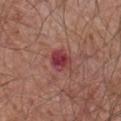Impression:
No biopsy was performed on this lesion — it was imaged during a full skin examination and was not determined to be concerning.
Image and clinical context:
The lesion is on the chest. The total-body-photography lesion software estimated an average lesion color of about L≈40 a*≈32 b*≈21 (CIELAB) and a normalized border contrast of about 9.5. And it measured a nevus-likeness score of about 0/100 and a lesion-detection confidence of about 100/100. A male patient, aged 63 to 67. The tile uses white-light illumination. A roughly 15 mm field-of-view crop from a total-body skin photograph. About 3 mm across.